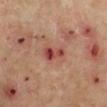Findings:
- notes: no biopsy performed (imaged during a skin exam)
- tile lighting: cross-polarized illumination
- diameter: ~3.5 mm (longest diameter)
- image-analysis metrics: a mean CIELAB color near L≈45 a*≈31 b*≈27, roughly 12 lightness units darker than nearby skin, and a lesion-to-skin contrast of about 9 (normalized; higher = more distinct)
- acquisition: ~15 mm crop, total-body skin-cancer survey
- subject: male, aged 63 to 67
- anatomic site: the left lower leg